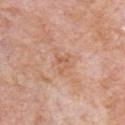No biopsy was performed on this lesion — it was imaged during a full skin examination and was not determined to be concerning. On the chest. The lesion-visualizer software estimated an eccentricity of roughly 0.55. The software also gave a color-variation rating of about 4/10 and a peripheral color-asymmetry measure near 1.5. The subject is a male aged 78 to 82. This image is a 15 mm lesion crop taken from a total-body photograph. Captured under white-light illumination.